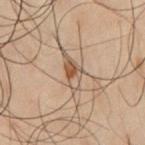Part of a total-body skin-imaging series; this lesion was reviewed on a skin check and was not flagged for biopsy. Longest diameter approximately 4 mm. A region of skin cropped from a whole-body photographic capture, roughly 15 mm wide. The lesion is on the chest. The subject is a male aged 48 to 52. This is a cross-polarized tile.